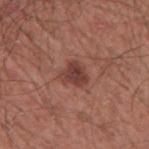The total-body-photography lesion software estimated roughly 10 lightness units darker than nearby skin and a lesion-to-skin contrast of about 8.5 (normalized; higher = more distinct). The analysis additionally found an automated nevus-likeness rating near 50 out of 100 and a detector confidence of about 100 out of 100 that the crop contains a lesion. On the arm. Captured under white-light illumination. The patient is a male about 60 years old. A 15 mm crop from a total-body photograph taken for skin-cancer surveillance. Longest diameter approximately 3 mm.